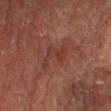Case summary:
- follow-up — imaged on a skin check; not biopsied
- lighting — cross-polarized illumination
- image — ~15 mm crop, total-body skin-cancer survey
- location — the right thigh
- subject — female
- size — about 4.5 mm
- automated metrics — a lesion color around L≈39 a*≈26 b*≈28 in CIELAB, roughly 6 lightness units darker than nearby skin, and a normalized border contrast of about 5.5; an automated nevus-likeness rating near 0 out of 100 and a lesion-detection confidence of about 95/100